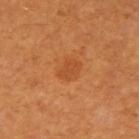Part of a total-body skin-imaging series; this lesion was reviewed on a skin check and was not flagged for biopsy. Longest diameter approximately 2.5 mm. A male patient, in their 60s. The lesion-visualizer software estimated an area of roughly 4.5 mm² and a symmetry-axis asymmetry near 0.3. The software also gave an average lesion color of about L≈44 a*≈27 b*≈39 (CIELAB), about 6 CIELAB-L* units darker than the surrounding skin, and a lesion-to-skin contrast of about 5 (normalized; higher = more distinct). The software also gave border irregularity of about 2.5 on a 0–10 scale, internal color variation of about 1.5 on a 0–10 scale, and radial color variation of about 0.5. A 15 mm close-up extracted from a 3D total-body photography capture. Located on the right upper arm. Imaged with cross-polarized lighting.